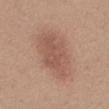Impression:
The lesion was photographed on a routine skin check and not biopsied; there is no pathology result.
Image and clinical context:
A lesion tile, about 15 mm wide, cut from a 3D total-body photograph. Longest diameter approximately 7 mm. Located on the mid back. The patient is a male roughly 30 years of age. The tile uses white-light illumination.